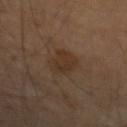Impression:
Part of a total-body skin-imaging series; this lesion was reviewed on a skin check and was not flagged for biopsy.
Clinical summary:
The lesion-visualizer software estimated a footprint of about 7.5 mm², an outline eccentricity of about 0.75 (0 = round, 1 = elongated), and two-axis asymmetry of about 0.2. A male patient roughly 65 years of age. The lesion is on the arm. A close-up tile cropped from a whole-body skin photograph, about 15 mm across. Approximately 4 mm at its widest. Imaged with cross-polarized lighting.